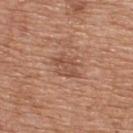Part of a total-body skin-imaging series; this lesion was reviewed on a skin check and was not flagged for biopsy. A male subject, in their mid- to late 60s. Located on the upper back. Automated tile analysis of the lesion measured a mean CIELAB color near L≈51 a*≈22 b*≈30 and a lesion–skin lightness drop of about 8. Captured under white-light illumination. About 4 mm across. A roughly 15 mm field-of-view crop from a total-body skin photograph.